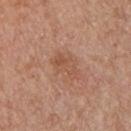Captured during whole-body skin photography for melanoma surveillance; the lesion was not biopsied.
Imaged with white-light lighting.
A male patient approximately 55 years of age.
Located on the front of the torso.
Cropped from a total-body skin-imaging series; the visible field is about 15 mm.